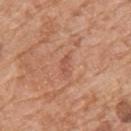Captured during whole-body skin photography for melanoma surveillance; the lesion was not biopsied.
Cropped from a total-body skin-imaging series; the visible field is about 15 mm.
A male subject, roughly 60 years of age.
From the upper back.